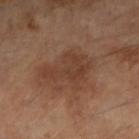{
  "biopsy_status": "not biopsied; imaged during a skin examination",
  "image": {
    "source": "total-body photography crop",
    "field_of_view_mm": 15
  },
  "lesion_size": {
    "long_diameter_mm_approx": 5.0
  },
  "patient": {
    "sex": "female",
    "age_approx": 60
  },
  "site": "right forearm",
  "lighting": "cross-polarized",
  "automated_metrics": {
    "area_mm2_approx": 14.0,
    "shape_asymmetry": 0.45,
    "cielab_L": 40,
    "cielab_a": 20,
    "cielab_b": 29,
    "vs_skin_darker_L": 7.0,
    "vs_skin_contrast_norm": 6.0,
    "border_irregularity_0_10": 6.0,
    "peripheral_color_asymmetry": 1.0,
    "nevus_likeness_0_100": 0,
    "lesion_detection_confidence_0_100": 100
  }
}